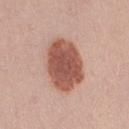Part of a total-body skin-imaging series; this lesion was reviewed on a skin check and was not flagged for biopsy. Approximately 6.5 mm at its widest. Located on the right thigh. A female subject aged 23–27. This is a white-light tile. A 15 mm close-up tile from a total-body photography series done for melanoma screening.